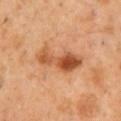The lesion was tiled from a total-body skin photograph and was not biopsied. The subject is a male aged 48 to 52. A close-up tile cropped from a whole-body skin photograph, about 15 mm across. Imaged with cross-polarized lighting. On the chest.